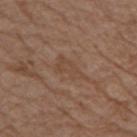lesion size: about 4 mm
acquisition: ~15 mm crop, total-body skin-cancer survey
subject: female, aged 83 or older
site: the left upper arm
illumination: white-light